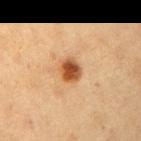{"biopsy_status": "not biopsied; imaged during a skin examination", "image": {"source": "total-body photography crop", "field_of_view_mm": 15}, "site": "right upper arm", "lesion_size": {"long_diameter_mm_approx": 3.0}, "patient": {"sex": "female", "age_approx": 40}, "lighting": "cross-polarized", "automated_metrics": {"area_mm2_approx": 5.5, "eccentricity": 0.6, "shape_asymmetry": 0.15, "border_irregularity_0_10": 1.5, "color_variation_0_10": 5.0, "peripheral_color_asymmetry": 1.5, "nevus_likeness_0_100": 100, "lesion_detection_confidence_0_100": 100}}